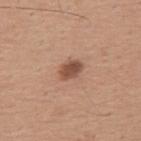Q: Was this lesion biopsied?
A: imaged on a skin check; not biopsied
Q: Lesion location?
A: the upper back
Q: Who is the patient?
A: male, approximately 65 years of age
Q: How was the tile lit?
A: white-light
Q: Lesion size?
A: ≈3 mm
Q: How was this image acquired?
A: ~15 mm tile from a whole-body skin photo